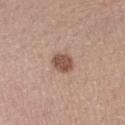Notes:
• follow-up · no biopsy performed (imaged during a skin exam)
• acquisition · 15 mm crop, total-body photography
• lighting · white-light illumination
• site · the left forearm
• patient · female, aged 28–32
• automated metrics · an average lesion color of about L≈51 a*≈20 b*≈26 (CIELAB) and a normalized lesion–skin contrast near 9; a nevus-likeness score of about 90/100 and a detector confidence of about 100 out of 100 that the crop contains a lesion
• lesion diameter · ≈2.5 mm